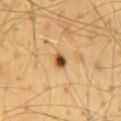The lesion was photographed on a routine skin check and not biopsied; there is no pathology result.
About 2 mm across.
The subject is a male roughly 65 years of age.
The tile uses cross-polarized illumination.
This image is a 15 mm lesion crop taken from a total-body photograph.
Automated image analysis of the tile measured a shape eccentricity near 0.65 and two-axis asymmetry of about 0.25.
From the mid back.